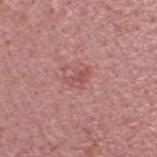This lesion was catalogued during total-body skin photography and was not selected for biopsy.
Automated image analysis of the tile measured a footprint of about 3 mm², a shape eccentricity near 0.85, and two-axis asymmetry of about 0.55. And it measured a normalized lesion–skin contrast near 5.5. It also reported a classifier nevus-likeness of about 0/100 and lesion-presence confidence of about 100/100.
A male patient, aged approximately 35.
The lesion's longest dimension is about 3 mm.
On the head or neck.
A 15 mm close-up extracted from a 3D total-body photography capture.